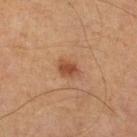| key | value |
|---|---|
| biopsy status | catalogued during a skin exam; not biopsied |
| tile lighting | cross-polarized illumination |
| acquisition | 15 mm crop, total-body photography |
| lesion size | about 2.5 mm |
| image-analysis metrics | an automated nevus-likeness rating near 90 out of 100 and a lesion-detection confidence of about 100/100 |
| anatomic site | the right lower leg |
| subject | aged 53–57 |